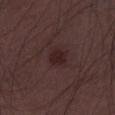workup: catalogued during a skin exam; not biopsied
site: the leg
subject: male, approximately 50 years of age
acquisition: 15 mm crop, total-body photography